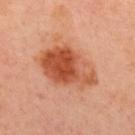| key | value |
|---|---|
| follow-up | no biopsy performed (imaged during a skin exam) |
| subject | male, roughly 50 years of age |
| lesion size | ~7 mm (longest diameter) |
| image | ~15 mm tile from a whole-body skin photo |
| site | the upper back |